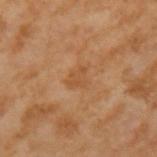No biopsy was performed on this lesion — it was imaged during a full skin examination and was not determined to be concerning. The patient is a male aged approximately 65. Measured at roughly 3 mm in maximum diameter. A region of skin cropped from a whole-body photographic capture, roughly 15 mm wide.